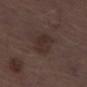follow-up = catalogued during a skin exam; not biopsied
patient = male, aged 68 to 72
location = the left thigh
image = ~15 mm tile from a whole-body skin photo